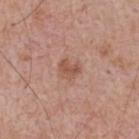Clinical impression:
Captured during whole-body skin photography for melanoma surveillance; the lesion was not biopsied.
Image and clinical context:
A roughly 15 mm field-of-view crop from a total-body skin photograph. Automated tile analysis of the lesion measured an area of roughly 4.5 mm² and a shape-asymmetry score of about 0.3 (0 = symmetric). It also reported an average lesion color of about L≈53 a*≈22 b*≈29 (CIELAB). The software also gave a border-irregularity rating of about 3/10, internal color variation of about 2 on a 0–10 scale, and a peripheral color-asymmetry measure near 1. It also reported a classifier nevus-likeness of about 5/100. The patient is a male aged 63–67. The lesion's longest dimension is about 2.5 mm. The lesion is on the upper back.